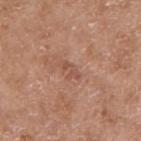notes — catalogued during a skin exam; not biopsied
site — the right upper arm
subject — female, roughly 75 years of age
size — ≈2.5 mm
tile lighting — white-light illumination
imaging modality — total-body-photography crop, ~15 mm field of view